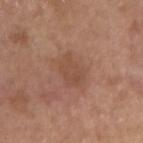workup: no biopsy performed (imaged during a skin exam) | patient: female, aged approximately 65 | lesion size: ≈3.5 mm | acquisition: 15 mm crop, total-body photography | image-analysis metrics: an average lesion color of about L≈48 a*≈20 b*≈29 (CIELAB) and a lesion-to-skin contrast of about 5 (normalized; higher = more distinct); a nevus-likeness score of about 0/100 and a lesion-detection confidence of about 100/100 | illumination: white-light | location: the right upper arm.